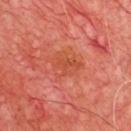Assessment:
Part of a total-body skin-imaging series; this lesion was reviewed on a skin check and was not flagged for biopsy.
Clinical summary:
Imaged with cross-polarized lighting. Cropped from a whole-body photographic skin survey; the tile spans about 15 mm. An algorithmic analysis of the crop reported a lesion color around L≈50 a*≈34 b*≈37 in CIELAB, a lesion–skin lightness drop of about 6, and a lesion-to-skin contrast of about 5.5 (normalized; higher = more distinct). The software also gave a classifier nevus-likeness of about 0/100 and a lesion-detection confidence of about 100/100. The patient is a male aged 63 to 67. From the chest. About 3.5 mm across.